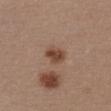No biopsy was performed on this lesion — it was imaged during a full skin examination and was not determined to be concerning.
The subject is a female in their 30s.
The lesion is on the chest.
The tile uses white-light illumination.
The lesion-visualizer software estimated two-axis asymmetry of about 0.25. It also reported a mean CIELAB color near L≈45 a*≈20 b*≈28, a lesion–skin lightness drop of about 11, and a normalized lesion–skin contrast near 9. The analysis additionally found a border-irregularity rating of about 2/10, a within-lesion color-variation index near 3/10, and radial color variation of about 1. It also reported a classifier nevus-likeness of about 90/100.
A 15 mm close-up tile from a total-body photography series done for melanoma screening.
Approximately 2.5 mm at its widest.